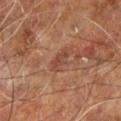| field | value |
|---|---|
| biopsy status | catalogued during a skin exam; not biopsied |
| lesion size | about 3 mm |
| image source | 15 mm crop, total-body photography |
| site | the right leg |
| subject | male, about 60 years old |